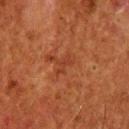{
  "biopsy_status": "not biopsied; imaged during a skin examination",
  "image": {
    "source": "total-body photography crop",
    "field_of_view_mm": 15
  },
  "automated_metrics": {
    "border_irregularity_0_10": 5.5,
    "color_variation_0_10": 0.0,
    "peripheral_color_asymmetry": 0.0
  },
  "patient": {
    "sex": "male",
    "age_approx": 65
  },
  "lesion_size": {
    "long_diameter_mm_approx": 2.5
  },
  "site": "head or neck",
  "lighting": "cross-polarized"
}